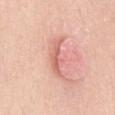Acquisition and patient details: The lesion-visualizer software estimated a footprint of about 6.5 mm², a shape eccentricity near 0.95, and a symmetry-axis asymmetry near 0.4. It also reported a nevus-likeness score of about 0/100 and a detector confidence of about 90 out of 100 that the crop contains a lesion. On the lower back. Longest diameter approximately 4.5 mm. Cropped from a total-body skin-imaging series; the visible field is about 15 mm. The patient is a male in their mid- to late 50s. Captured under white-light illumination.